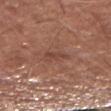Notes:
– follow-up: catalogued during a skin exam; not biopsied
– body site: the left forearm
– lighting: white-light illumination
– lesion size: ~2.5 mm (longest diameter)
– acquisition: ~15 mm tile from a whole-body skin photo
– patient: male, about 65 years old